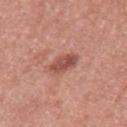Q: Was this lesion biopsied?
A: catalogued during a skin exam; not biopsied
Q: What kind of image is this?
A: 15 mm crop, total-body photography
Q: Illumination type?
A: white-light
Q: Patient demographics?
A: female, aged approximately 40
Q: Where on the body is the lesion?
A: the left upper arm
Q: What did automated image analysis measure?
A: a mean CIELAB color near L≈51 a*≈26 b*≈27, roughly 12 lightness units darker than nearby skin, and a normalized lesion–skin contrast near 8; a nevus-likeness score of about 40/100 and lesion-presence confidence of about 100/100